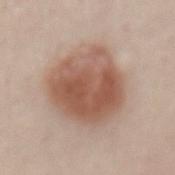Part of a total-body skin-imaging series; this lesion was reviewed on a skin check and was not flagged for biopsy. The lesion is located on the lower back. Captured under white-light illumination. A male subject, about 65 years old. A 15 mm close-up extracted from a 3D total-body photography capture.